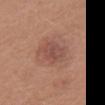Case summary:
• biopsy status: catalogued during a skin exam; not biopsied
• subject: female, aged around 50
• tile lighting: white-light illumination
• body site: the head or neck
• diameter: about 4.5 mm
• automated metrics: an outline eccentricity of about 0.8 (0 = round, 1 = elongated) and a symmetry-axis asymmetry near 0.2; a mean CIELAB color near L≈50 a*≈22 b*≈26, roughly 8 lightness units darker than nearby skin, and a normalized border contrast of about 6
• image source: total-body-photography crop, ~15 mm field of view